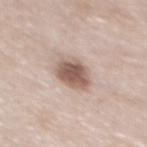<lesion>
  <patient>
    <sex>female</sex>
    <age_approx>65</age_approx>
  </patient>
  <lesion_size>
    <long_diameter_mm_approx>3.5</long_diameter_mm_approx>
  </lesion_size>
  <lighting>white-light</lighting>
  <site>back</site>
  <automated_metrics>
    <cielab_L>56</cielab_L>
    <cielab_a>17</cielab_a>
    <cielab_b>24</cielab_b>
    <vs_skin_darker_L>15.0</vs_skin_darker_L>
    <vs_skin_contrast_norm>10.0</vs_skin_contrast_norm>
    <nevus_likeness_0_100>95</nevus_likeness_0_100>
    <lesion_detection_confidence_0_100>100</lesion_detection_confidence_0_100>
  </automated_metrics>
  <image>
    <source>total-body photography crop</source>
    <field_of_view_mm>15</field_of_view_mm>
  </image>
</lesion>